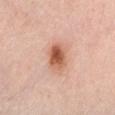<case>
  <biopsy_status>not biopsied; imaged during a skin examination</biopsy_status>
  <lesion_size>
    <long_diameter_mm_approx>3.5</long_diameter_mm_approx>
  </lesion_size>
  <image>
    <source>total-body photography crop</source>
    <field_of_view_mm>15</field_of_view_mm>
  </image>
  <lighting>white-light</lighting>
  <patient>
    <sex>female</sex>
    <age_approx>65</age_approx>
  </patient>
  <site>chest</site>
</case>